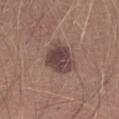<record>
<biopsy_status>not biopsied; imaged during a skin examination</biopsy_status>
</record>